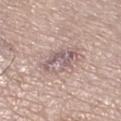Assessment:
The lesion was tiled from a total-body skin photograph and was not biopsied.
Image and clinical context:
The patient is a male aged 68 to 72. The lesion is on the leg. Longest diameter approximately 4 mm. A roughly 15 mm field-of-view crop from a total-body skin photograph. Captured under white-light illumination.